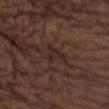{
  "biopsy_status": "not biopsied; imaged during a skin examination",
  "automated_metrics": {
    "area_mm2_approx": 4.0,
    "eccentricity": 0.8,
    "shape_asymmetry": 0.55,
    "cielab_L": 26,
    "cielab_a": 17,
    "cielab_b": 21,
    "vs_skin_darker_L": 5.0,
    "vs_skin_contrast_norm": 6.0,
    "nevus_likeness_0_100": 0,
    "lesion_detection_confidence_0_100": 70
  },
  "site": "left lower leg",
  "image": {
    "source": "total-body photography crop",
    "field_of_view_mm": 15
  },
  "lesion_size": {
    "long_diameter_mm_approx": 3.5
  },
  "patient": {
    "sex": "male",
    "age_approx": 75
  }
}